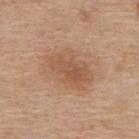Clinical impression: No biopsy was performed on this lesion — it was imaged during a full skin examination and was not determined to be concerning. Background: A male subject roughly 65 years of age. The tile uses white-light illumination. A 15 mm close-up tile from a total-body photography series done for melanoma screening. The lesion is on the upper back. The total-body-photography lesion software estimated a detector confidence of about 100 out of 100 that the crop contains a lesion. The lesion's longest dimension is about 5.5 mm.